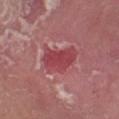The lesion was tiled from a total-body skin photograph and was not biopsied.
About 4.5 mm across.
A male patient, approximately 65 years of age.
Imaged with white-light lighting.
A region of skin cropped from a whole-body photographic capture, roughly 15 mm wide.
On the right lower leg.
Automated tile analysis of the lesion measured a lesion color around L≈45 a*≈35 b*≈20 in CIELAB and a lesion-to-skin contrast of about 7 (normalized; higher = more distinct). It also reported a border-irregularity index near 3/10, a within-lesion color-variation index near 2/10, and radial color variation of about 0.5. It also reported a nevus-likeness score of about 15/100 and a lesion-detection confidence of about 85/100.